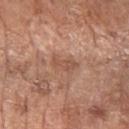Part of a total-body skin-imaging series; this lesion was reviewed on a skin check and was not flagged for biopsy. Captured under white-light illumination. Automated image analysis of the tile measured a footprint of about 4.5 mm² and a shape eccentricity near 0.8. The lesion's longest dimension is about 3 mm. The lesion is on the right forearm. A female subject, in their mid-70s. Cropped from a whole-body photographic skin survey; the tile spans about 15 mm.